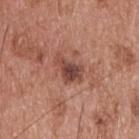biopsy_status: not biopsied; imaged during a skin examination
image:
  source: total-body photography crop
  field_of_view_mm: 15
lighting: white-light
patient:
  sex: male
  age_approx: 55
site: upper back